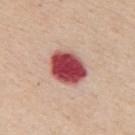Captured during whole-body skin photography for melanoma surveillance; the lesion was not biopsied.
The subject is a male roughly 65 years of age.
A 15 mm close-up tile from a total-body photography series done for melanoma screening.
From the mid back.